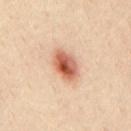This lesion was catalogued during total-body skin photography and was not selected for biopsy. Automated tile analysis of the lesion measured a mean CIELAB color near L≈58 a*≈25 b*≈33, roughly 16 lightness units darker than nearby skin, and a normalized border contrast of about 10. A male patient in their mid- to late 30s. From the mid back. A roughly 15 mm field-of-view crop from a total-body skin photograph. Captured under cross-polarized illumination.